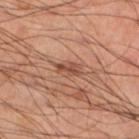notes — no biopsy performed (imaged during a skin exam)
subject — male, roughly 50 years of age
anatomic site — the left lower leg
lesion size — ≈3 mm
imaging modality — ~15 mm tile from a whole-body skin photo
automated lesion analysis — an area of roughly 4.5 mm², an eccentricity of roughly 0.85, and a shape-asymmetry score of about 0.3 (0 = symmetric); a mean CIELAB color near L≈50 a*≈23 b*≈30, roughly 9 lightness units darker than nearby skin, and a normalized border contrast of about 7; a nevus-likeness score of about 0/100 and lesion-presence confidence of about 95/100
lighting — cross-polarized